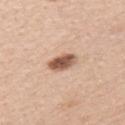notes = total-body-photography surveillance lesion; no biopsy | acquisition = 15 mm crop, total-body photography | subject = female, approximately 45 years of age | illumination = white-light illumination | TBP lesion metrics = a lesion color around L≈56 a*≈20 b*≈30 in CIELAB, about 17 CIELAB-L* units darker than the surrounding skin, and a normalized lesion–skin contrast near 10.5; a nevus-likeness score of about 95/100 and a detector confidence of about 100 out of 100 that the crop contains a lesion | body site = the left upper arm.